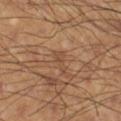• notes — imaged on a skin check; not biopsied
• diameter — about 3 mm
• image-analysis metrics — a footprint of about 3 mm², an eccentricity of roughly 0.9, and two-axis asymmetry of about 0.55; a lesion color around L≈46 a*≈19 b*≈30 in CIELAB and a lesion–skin lightness drop of about 6; a border-irregularity rating of about 6/10, a color-variation rating of about 0.5/10, and peripheral color asymmetry of about 0
• subject — male, in their 60s
• body site — the right lower leg
• image source — total-body-photography crop, ~15 mm field of view
• tile lighting — cross-polarized